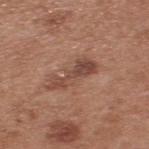Notes:
* follow-up: imaged on a skin check; not biopsied
* body site: the back
* illumination: white-light illumination
* size: ~5.5 mm (longest diameter)
* patient: male, aged around 55
* acquisition: ~15 mm tile from a whole-body skin photo